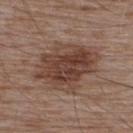| field | value |
|---|---|
| workup | catalogued during a skin exam; not biopsied |
| anatomic site | the upper back |
| TBP lesion metrics | an average lesion color of about L≈42 a*≈18 b*≈25 (CIELAB) and about 11 CIELAB-L* units darker than the surrounding skin |
| diameter | ≈7.5 mm |
| subject | male, aged 53 to 57 |
| image | 15 mm crop, total-body photography |
| lighting | white-light illumination |